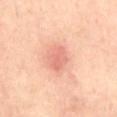Part of a total-body skin-imaging series; this lesion was reviewed on a skin check and was not flagged for biopsy.
Longest diameter approximately 3 mm.
The total-body-photography lesion software estimated a footprint of about 5 mm². And it measured an average lesion color of about L≈66 a*≈28 b*≈31 (CIELAB), about 9 CIELAB-L* units darker than the surrounding skin, and a normalized lesion–skin contrast near 5.5. And it measured border irregularity of about 2 on a 0–10 scale and a peripheral color-asymmetry measure near 1. The software also gave a nevus-likeness score of about 50/100 and a lesion-detection confidence of about 100/100.
Located on the front of the torso.
A male subject aged 68–72.
Cropped from a whole-body photographic skin survey; the tile spans about 15 mm.